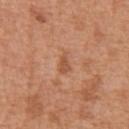Clinical impression:
The lesion was photographed on a routine skin check and not biopsied; there is no pathology result.
Acquisition and patient details:
On the abdomen. A male subject roughly 65 years of age. A close-up tile cropped from a whole-body skin photograph, about 15 mm across.